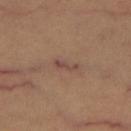Part of a total-body skin-imaging series; this lesion was reviewed on a skin check and was not flagged for biopsy.
A female subject in their 60s.
The lesion is located on the leg.
A 15 mm close-up extracted from a 3D total-body photography capture.